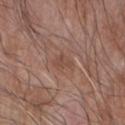biopsy status = no biopsy performed (imaged during a skin exam) | size = about 3 mm | subject = male, roughly 60 years of age | illumination = white-light illumination | anatomic site = the right forearm | image source = 15 mm crop, total-body photography.